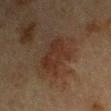The lesion was photographed on a routine skin check and not biopsied; there is no pathology result.
The patient is a male about 65 years old.
Captured under cross-polarized illumination.
Automated image analysis of the tile measured an area of roughly 14 mm². The software also gave a border-irregularity index near 4.5/10, a color-variation rating of about 2.5/10, and peripheral color asymmetry of about 1. The software also gave an automated nevus-likeness rating near 35 out of 100 and a lesion-detection confidence of about 100/100.
From the right upper arm.
A roughly 15 mm field-of-view crop from a total-body skin photograph.
The lesion's longest dimension is about 5 mm.